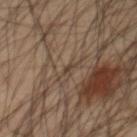follow-up: catalogued during a skin exam; not biopsied | patient: male, roughly 60 years of age | imaging modality: ~15 mm crop, total-body skin-cancer survey | anatomic site: the chest | tile lighting: cross-polarized illumination.